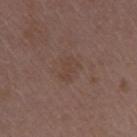imaging modality: ~15 mm tile from a whole-body skin photo | lesion size: ~2.5 mm (longest diameter) | patient: female, in their mid- to late 40s | automated lesion analysis: a lesion area of about 4.5 mm², a shape eccentricity near 0.75, and a symmetry-axis asymmetry near 0.3; an average lesion color of about L≈41 a*≈16 b*≈24 (CIELAB) and a lesion–skin lightness drop of about 4 | body site: the right upper arm | lighting: white-light illumination.